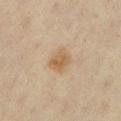Imaged during a routine full-body skin examination; the lesion was not biopsied and no histopathology is available.